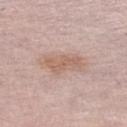notes: catalogued during a skin exam; not biopsied | site: the left lower leg | subject: female, aged around 65 | image: total-body-photography crop, ~15 mm field of view.